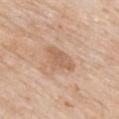Background: The lesion is on the upper back. Automated tile analysis of the lesion measured an area of roughly 7 mm² and a shape-asymmetry score of about 0.4 (0 = symmetric). And it measured about 8 CIELAB-L* units darker than the surrounding skin and a normalized border contrast of about 6. And it measured a detector confidence of about 100 out of 100 that the crop contains a lesion. A male subject, aged around 85 or older. A region of skin cropped from a whole-body photographic capture, roughly 15 mm wide. The lesion's longest dimension is about 4 mm.